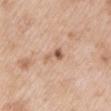| key | value |
|---|---|
| workup | total-body-photography surveillance lesion; no biopsy |
| lesion size | ≈3 mm |
| image-analysis metrics | a footprint of about 3.5 mm², an outline eccentricity of about 0.85 (0 = round, 1 = elongated), and a symmetry-axis asymmetry near 0.5; an average lesion color of about L≈60 a*≈20 b*≈31 (CIELAB), about 11 CIELAB-L* units darker than the surrounding skin, and a normalized lesion–skin contrast near 7 |
| imaging modality | ~15 mm tile from a whole-body skin photo |
| subject | female, in their 40s |
| illumination | white-light |
| body site | the arm |